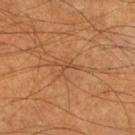Findings:
* follow-up · total-body-photography surveillance lesion; no biopsy
* acquisition · total-body-photography crop, ~15 mm field of view
* patient · male, aged 63 to 67
* body site · the right lower leg